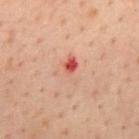The lesion was photographed on a routine skin check and not biopsied; there is no pathology result.
Longest diameter approximately 3.5 mm.
From the mid back.
The total-body-photography lesion software estimated a footprint of about 6 mm², a shape eccentricity near 0.55, and two-axis asymmetry of about 0.4. And it measured a mean CIELAB color near L≈48 a*≈22 b*≈26, roughly 7 lightness units darker than nearby skin, and a lesion-to-skin contrast of about 6 (normalized; higher = more distinct).
This image is a 15 mm lesion crop taken from a total-body photograph.
A male patient, about 40 years old.